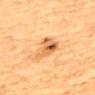The lesion was tiled from a total-body skin photograph and was not biopsied. Automated image analysis of the tile measured an average lesion color of about L≈57 a*≈21 b*≈40 (CIELAB), roughly 11 lightness units darker than nearby skin, and a normalized border contrast of about 7.5. The analysis additionally found a border-irregularity index near 5.5/10, a within-lesion color-variation index near 9.5/10, and peripheral color asymmetry of about 3.5. The software also gave an automated nevus-likeness rating near 40 out of 100 and a detector confidence of about 100 out of 100 that the crop contains a lesion. This is a cross-polarized tile. A male subject in their mid-80s or older. From the upper back. Longest diameter approximately 5 mm. Cropped from a whole-body photographic skin survey; the tile spans about 15 mm.